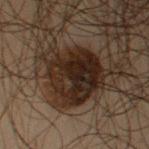Notes:
- acquisition: 15 mm crop, total-body photography
- patient: male, aged 48–52
- illumination: cross-polarized illumination
- TBP lesion metrics: an automated nevus-likeness rating near 30 out of 100 and a detector confidence of about 100 out of 100 that the crop contains a lesion
- body site: the left upper arm
- diameter: ~7.5 mm (longest diameter)